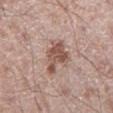This lesion was catalogued during total-body skin photography and was not selected for biopsy.
From the right lower leg.
About 5 mm across.
Captured under white-light illumination.
A close-up tile cropped from a whole-body skin photograph, about 15 mm across.
A male subject, aged 33–37.